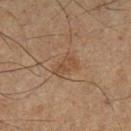Q: Was a biopsy performed?
A: total-body-photography surveillance lesion; no biopsy
Q: What did automated image analysis measure?
A: border irregularity of about 5.5 on a 0–10 scale, a color-variation rating of about 0/10, and radial color variation of about 0
Q: Patient demographics?
A: male, aged 63 to 67
Q: How was the tile lit?
A: cross-polarized illumination
Q: What is the lesion's diameter?
A: ~3 mm (longest diameter)
Q: How was this image acquired?
A: 15 mm crop, total-body photography
Q: Where on the body is the lesion?
A: the leg